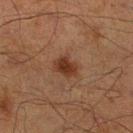Part of a total-body skin-imaging series; this lesion was reviewed on a skin check and was not flagged for biopsy. Imaged with cross-polarized lighting. A male subject roughly 70 years of age. The lesion is located on the right lower leg. The lesion's longest dimension is about 3 mm. A lesion tile, about 15 mm wide, cut from a 3D total-body photograph. Automated tile analysis of the lesion measured a mean CIELAB color near L≈29 a*≈19 b*≈26, about 9 CIELAB-L* units darker than the surrounding skin, and a normalized border contrast of about 9. It also reported border irregularity of about 2.5 on a 0–10 scale and a peripheral color-asymmetry measure near 0.5. And it measured an automated nevus-likeness rating near 95 out of 100 and a lesion-detection confidence of about 100/100.